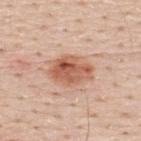biopsy_status: not biopsied; imaged during a skin examination
image:
  source: total-body photography crop
  field_of_view_mm: 15
site: upper back
lesion_size:
  long_diameter_mm_approx: 5.0
patient:
  sex: male
  age_approx: 50
lighting: white-light